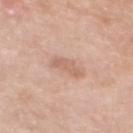Clinical summary:
A female patient, aged approximately 65. About 4 mm across. Automated tile analysis of the lesion measured an area of roughly 5.5 mm² and an outline eccentricity of about 0.9 (0 = round, 1 = elongated). The analysis additionally found a color-variation rating of about 1.5/10 and a peripheral color-asymmetry measure near 0.5. And it measured an automated nevus-likeness rating near 0 out of 100 and lesion-presence confidence of about 100/100. Captured under white-light illumination. On the mid back. A region of skin cropped from a whole-body photographic capture, roughly 15 mm wide.